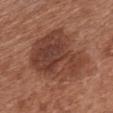{
  "biopsy_status": "not biopsied; imaged during a skin examination",
  "patient": {
    "sex": "female",
    "age_approx": 65
  },
  "site": "chest",
  "image": {
    "source": "total-body photography crop",
    "field_of_view_mm": 15
  }
}